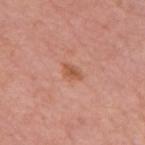Notes:
- workup · catalogued during a skin exam; not biopsied
- automated metrics · a shape eccentricity near 0.8 and a shape-asymmetry score of about 0.3 (0 = symmetric); a lesion color around L≈55 a*≈25 b*≈34 in CIELAB, roughly 9 lightness units darker than nearby skin, and a normalized lesion–skin contrast near 7; a border-irregularity index near 2.5/10 and a color-variation rating of about 1.5/10
- lesion diameter · ~2.5 mm (longest diameter)
- tile lighting · white-light
- patient · male, in their mid-70s
- anatomic site · the mid back
- acquisition · ~15 mm crop, total-body skin-cancer survey